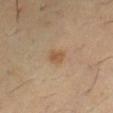Assessment:
The lesion was tiled from a total-body skin photograph and was not biopsied.
Clinical summary:
About 2 mm across. A male patient aged approximately 60. Automated image analysis of the tile measured a footprint of about 3 mm², an outline eccentricity of about 0.7 (0 = round, 1 = elongated), and a shape-asymmetry score of about 0.3 (0 = symmetric). It also reported a lesion–skin lightness drop of about 8 and a normalized lesion–skin contrast near 6.5. The analysis additionally found a classifier nevus-likeness of about 65/100 and a lesion-detection confidence of about 100/100. From the right thigh. A lesion tile, about 15 mm wide, cut from a 3D total-body photograph. This is a cross-polarized tile.